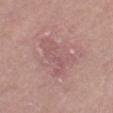Q: Is there a histopathology result?
A: no biopsy performed (imaged during a skin exam)
Q: What is the anatomic site?
A: the right thigh
Q: What lighting was used for the tile?
A: white-light
Q: What is the imaging modality?
A: 15 mm crop, total-body photography
Q: How large is the lesion?
A: about 5.5 mm
Q: Who is the patient?
A: male, aged approximately 75
Q: Automated lesion metrics?
A: a footprint of about 16 mm², an eccentricity of roughly 0.7, and two-axis asymmetry of about 0.45; a lesion color around L≈56 a*≈22 b*≈19 in CIELAB and a normalized border contrast of about 4.5